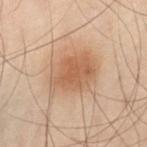This lesion was catalogued during total-body skin photography and was not selected for biopsy. Captured under cross-polarized illumination. The total-body-photography lesion software estimated a lesion color around L≈48 a*≈17 b*≈28 in CIELAB, a lesion–skin lightness drop of about 8, and a lesion-to-skin contrast of about 7 (normalized; higher = more distinct). And it measured a peripheral color-asymmetry measure near 1. The software also gave lesion-presence confidence of about 100/100. The lesion's longest dimension is about 6 mm. On the abdomen. The patient is a male aged around 50. A lesion tile, about 15 mm wide, cut from a 3D total-body photograph.